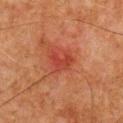Captured during whole-body skin photography for melanoma surveillance; the lesion was not biopsied. Imaged with cross-polarized lighting. The lesion is on the chest. A 15 mm close-up tile from a total-body photography series done for melanoma screening. The subject is a male about 80 years old. The lesion's longest dimension is about 3 mm.